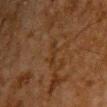Q: Was this lesion biopsied?
A: no biopsy performed (imaged during a skin exam)
Q: Who is the patient?
A: male, approximately 60 years of age
Q: What did automated image analysis measure?
A: a mean CIELAB color near L≈25 a*≈14 b*≈26 and roughly 4 lightness units darker than nearby skin
Q: How large is the lesion?
A: ~3 mm (longest diameter)
Q: How was this image acquired?
A: total-body-photography crop, ~15 mm field of view
Q: What is the anatomic site?
A: the chest